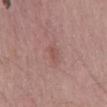About 2.5 mm across. Cropped from a total-body skin-imaging series; the visible field is about 15 mm. A male patient, approximately 55 years of age. Captured under white-light illumination. An algorithmic analysis of the crop reported a mean CIELAB color near L≈52 a*≈22 b*≈24, about 6 CIELAB-L* units darker than the surrounding skin, and a lesion-to-skin contrast of about 5 (normalized; higher = more distinct). The software also gave border irregularity of about 2.5 on a 0–10 scale.